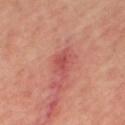Findings:
* notes · catalogued during a skin exam; not biopsied
* site · the right thigh
* imaging modality · ~15 mm tile from a whole-body skin photo
* subject · female, in their 50s